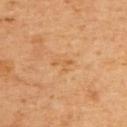Impression:
Imaged during a routine full-body skin examination; the lesion was not biopsied and no histopathology is available.
Background:
Approximately 2.5 mm at its widest. A male patient aged around 60. From the upper back. This image is a 15 mm lesion crop taken from a total-body photograph.